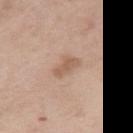diameter: about 3.5 mm
tile lighting: white-light
acquisition: ~15 mm tile from a whole-body skin photo
TBP lesion metrics: an eccentricity of roughly 0.85 and two-axis asymmetry of about 0.3; a within-lesion color-variation index near 2/10 and peripheral color asymmetry of about 0.5
body site: the leg
patient: female, roughly 55 years of age